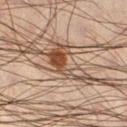Assessment: Imaged during a routine full-body skin examination; the lesion was not biopsied and no histopathology is available. Context: Captured under cross-polarized illumination. A close-up tile cropped from a whole-body skin photograph, about 15 mm across. The patient is a male aged approximately 40. From the left lower leg. Longest diameter approximately 6 mm.